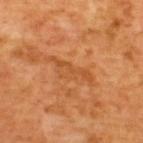<record>
  <biopsy_status>not biopsied; imaged during a skin examination</biopsy_status>
  <patient>
    <sex>male</sex>
    <age_approx>65</age_approx>
  </patient>
  <image>
    <source>total-body photography crop</source>
    <field_of_view_mm>15</field_of_view_mm>
  </image>
  <site>upper back</site>
  <lighting>cross-polarized</lighting>
</record>